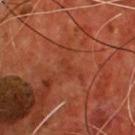Assessment:
No biopsy was performed on this lesion — it was imaged during a full skin examination and was not determined to be concerning.
Context:
A male patient aged 53–57. A 15 mm close-up tile from a total-body photography series done for melanoma screening. Located on the chest.